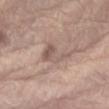Impression: Captured during whole-body skin photography for melanoma surveillance; the lesion was not biopsied. Image and clinical context: This is a white-light tile. A close-up tile cropped from a whole-body skin photograph, about 15 mm across. The lesion is on the arm. Approximately 4.5 mm at its widest. A male subject approximately 70 years of age.